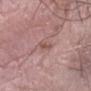The lesion was tiled from a total-body skin photograph and was not biopsied. Cropped from a total-body skin-imaging series; the visible field is about 15 mm. The patient is a male about 60 years old. On the left forearm. The lesion's longest dimension is about 3.5 mm. The total-body-photography lesion software estimated a lesion area of about 3 mm², a shape eccentricity near 0.95, and a symmetry-axis asymmetry near 0.3. The software also gave a border-irregularity index near 3.5/10 and peripheral color asymmetry of about 0. Captured under white-light illumination.